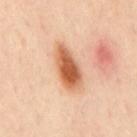| key | value |
|---|---|
| biopsy status | no biopsy performed (imaged during a skin exam) |
| acquisition | 15 mm crop, total-body photography |
| tile lighting | cross-polarized illumination |
| automated lesion analysis | a footprint of about 12 mm², a shape eccentricity near 0.9, and two-axis asymmetry of about 0.2; a border-irregularity rating of about 2/10 and a within-lesion color-variation index near 5.5/10; an automated nevus-likeness rating near 100 out of 100 and a detector confidence of about 100 out of 100 that the crop contains a lesion |
| patient | male, in their mid- to late 40s |
| body site | the mid back |
| size | ≈5.5 mm |